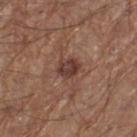| key | value |
|---|---|
| biopsy status | catalogued during a skin exam; not biopsied |
| body site | the left thigh |
| lesion size | ~2.5 mm (longest diameter) |
| image | ~15 mm crop, total-body skin-cancer survey |
| subject | male, in their mid-60s |
| illumination | white-light illumination |